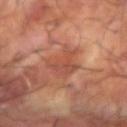The lesion was tiled from a total-body skin photograph and was not biopsied. Measured at roughly 4.5 mm in maximum diameter. Cropped from a whole-body photographic skin survey; the tile spans about 15 mm. A male subject, about 60 years old. From the right forearm. The tile uses cross-polarized illumination.